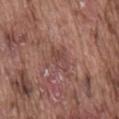Acquisition and patient details: A close-up tile cropped from a whole-body skin photograph, about 15 mm across. The lesion is located on the mid back. The subject is a male about 75 years old.